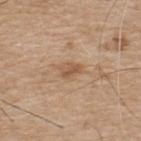Part of a total-body skin-imaging series; this lesion was reviewed on a skin check and was not flagged for biopsy.
Imaged with white-light lighting.
A male subject, aged 73–77.
The recorded lesion diameter is about 2.5 mm.
Automated tile analysis of the lesion measured an average lesion color of about L≈55 a*≈18 b*≈33 (CIELAB) and a normalized lesion–skin contrast near 6.5. And it measured a color-variation rating of about 3.5/10 and radial color variation of about 1.5.
On the upper back.
A 15 mm close-up tile from a total-body photography series done for melanoma screening.